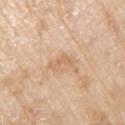This is a white-light tile.
Automated tile analysis of the lesion measured border irregularity of about 6 on a 0–10 scale and a within-lesion color-variation index near 0/10.
The lesion is located on the right upper arm.
This image is a 15 mm lesion crop taken from a total-body photograph.
Measured at roughly 2.5 mm in maximum diameter.
A male patient aged 78–82.